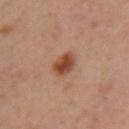biopsy status: no biopsy performed (imaged during a skin exam); image source: ~15 mm tile from a whole-body skin photo; body site: the left upper arm; subject: female, aged 38 to 42.